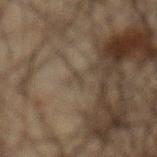Recorded during total-body skin imaging; not selected for excision or biopsy.
The lesion is on the abdomen.
An algorithmic analysis of the crop reported a footprint of about 1 mm², an eccentricity of roughly 0.95, and two-axis asymmetry of about 0.35.
A close-up tile cropped from a whole-body skin photograph, about 15 mm across.
A male subject roughly 65 years of age.
Imaged with cross-polarized lighting.
Longest diameter approximately 1.5 mm.